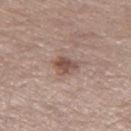biopsy status — total-body-photography surveillance lesion; no biopsy | lesion diameter — ~3 mm (longest diameter) | image source — ~15 mm tile from a whole-body skin photo | lighting — white-light | patient — female, in their mid- to late 60s | image-analysis metrics — a lesion–skin lightness drop of about 11 and a lesion-to-skin contrast of about 8 (normalized; higher = more distinct) | anatomic site — the left thigh.